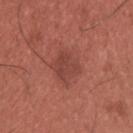The lesion was photographed on a routine skin check and not biopsied; there is no pathology result.
The lesion's longest dimension is about 4 mm.
A male patient about 35 years old.
The lesion is located on the upper back.
An algorithmic analysis of the crop reported a border-irregularity index near 2.5/10 and peripheral color asymmetry of about 1. And it measured an automated nevus-likeness rating near 10 out of 100 and a detector confidence of about 100 out of 100 that the crop contains a lesion.
A lesion tile, about 15 mm wide, cut from a 3D total-body photograph.
This is a white-light tile.